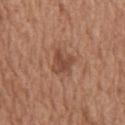lighting: white-light illumination
patient: male, in their mid-60s
site: the chest
image source: 15 mm crop, total-body photography
TBP lesion metrics: a mean CIELAB color near L≈47 a*≈22 b*≈29 and a lesion-to-skin contrast of about 7 (normalized; higher = more distinct); a within-lesion color-variation index near 2.5/10 and peripheral color asymmetry of about 0.5; a classifier nevus-likeness of about 0/100 and a detector confidence of about 100 out of 100 that the crop contains a lesion
size: about 3 mm